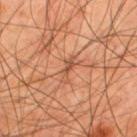| key | value |
|---|---|
| follow-up | no biopsy performed (imaged during a skin exam) |
| image-analysis metrics | a shape eccentricity near 0.95 and a shape-asymmetry score of about 0.4 (0 = symmetric) |
| imaging modality | ~15 mm tile from a whole-body skin photo |
| subject | male, aged 43 to 47 |
| lesion diameter | ~3.5 mm (longest diameter) |
| body site | the upper back |